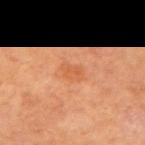{"biopsy_status": "not biopsied; imaged during a skin examination", "lesion_size": {"long_diameter_mm_approx": 2.5}, "patient": {"sex": "female", "age_approx": 65}, "image": {"source": "total-body photography crop", "field_of_view_mm": 15}, "lighting": "cross-polarized", "automated_metrics": {"shape_asymmetry": 0.3, "cielab_L": 56, "cielab_a": 28, "cielab_b": 40, "vs_skin_darker_L": 6.0, "vs_skin_contrast_norm": 5.0, "border_irregularity_0_10": 2.5, "color_variation_0_10": 2.0, "peripheral_color_asymmetry": 0.5, "nevus_likeness_0_100": 5, "lesion_detection_confidence_0_100": 100}, "site": "arm"}